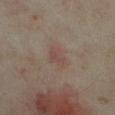Impression: No biopsy was performed on this lesion — it was imaged during a full skin examination and was not determined to be concerning. Background: The patient is a female roughly 35 years of age. A close-up tile cropped from a whole-body skin photograph, about 15 mm across. Captured under cross-polarized illumination. Approximately 2.5 mm at its widest. The lesion is located on the left lower leg. Automated image analysis of the tile measured an area of roughly 4.5 mm², a shape eccentricity near 0.6, and two-axis asymmetry of about 0.3. The software also gave an average lesion color of about L≈44 a*≈15 b*≈22 (CIELAB), a lesion–skin lightness drop of about 5, and a lesion-to-skin contrast of about 5 (normalized; higher = more distinct). It also reported a lesion-detection confidence of about 100/100.